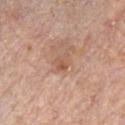biopsy_status: not biopsied; imaged during a skin examination
lighting: white-light
image:
  source: total-body photography crop
  field_of_view_mm: 15
automated_metrics:
  nevus_likeness_0_100: 5
  lesion_detection_confidence_0_100: 100
patient:
  sex: male
  age_approx: 60
site: chest